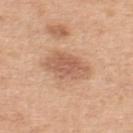Captured during whole-body skin photography for melanoma surveillance; the lesion was not biopsied. A region of skin cropped from a whole-body photographic capture, roughly 15 mm wide. The lesion is on the back. Automated tile analysis of the lesion measured an outline eccentricity of about 0.8 (0 = round, 1 = elongated) and a symmetry-axis asymmetry near 0.2. The analysis additionally found a lesion color around L≈59 a*≈22 b*≈32 in CIELAB, a lesion–skin lightness drop of about 11, and a normalized border contrast of about 7. Captured under white-light illumination. A male subject, approximately 50 years of age.